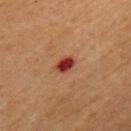This lesion was catalogued during total-body skin photography and was not selected for biopsy.
A male subject aged 53–57.
A roughly 15 mm field-of-view crop from a total-body skin photograph.
On the chest.
This is a cross-polarized tile.
About 2.5 mm across.